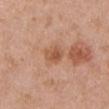Q: Was a biopsy performed?
A: imaged on a skin check; not biopsied
Q: What lighting was used for the tile?
A: white-light
Q: Where on the body is the lesion?
A: the left upper arm
Q: Patient demographics?
A: female, aged 48 to 52
Q: What is the imaging modality?
A: 15 mm crop, total-body photography